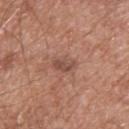{
  "biopsy_status": "not biopsied; imaged during a skin examination",
  "site": "back",
  "image": {
    "source": "total-body photography crop",
    "field_of_view_mm": 15
  },
  "lighting": "white-light",
  "patient": {
    "sex": "male",
    "age_approx": 65
  },
  "lesion_size": {
    "long_diameter_mm_approx": 3.0
  },
  "automated_metrics": {
    "area_mm2_approx": 4.0,
    "eccentricity": 0.8,
    "shape_asymmetry": 0.25,
    "cielab_L": 49,
    "cielab_a": 21,
    "cielab_b": 27,
    "vs_skin_darker_L": 8.0,
    "vs_skin_contrast_norm": 6.0,
    "color_variation_0_10": 3.0,
    "nevus_likeness_0_100": 0,
    "lesion_detection_confidence_0_100": 100
  }
}